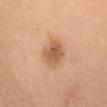Clinical impression: No biopsy was performed on this lesion — it was imaged during a full skin examination and was not determined to be concerning. Acquisition and patient details: Measured at roughly 3 mm in maximum diameter. This is a cross-polarized tile. A lesion tile, about 15 mm wide, cut from a 3D total-body photograph. A female patient, roughly 30 years of age. Located on the head or neck. The lesion-visualizer software estimated an outline eccentricity of about 0.45 (0 = round, 1 = elongated) and a symmetry-axis asymmetry near 0.2. The analysis additionally found an average lesion color of about L≈53 a*≈20 b*≈33 (CIELAB), roughly 11 lightness units darker than nearby skin, and a normalized border contrast of about 7.5. The software also gave a classifier nevus-likeness of about 80/100 and lesion-presence confidence of about 100/100.